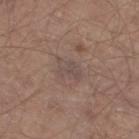* biopsy status — imaged on a skin check; not biopsied
* imaging modality — 15 mm crop, total-body photography
* automated metrics — a lesion color around L≈47 a*≈14 b*≈20 in CIELAB and roughly 5 lightness units darker than nearby skin; a border-irregularity rating of about 4/10, a color-variation rating of about 2.5/10, and peripheral color asymmetry of about 1; a detector confidence of about 95 out of 100 that the crop contains a lesion
* size — ≈3.5 mm
* location — the right thigh
* patient — male, in their mid-60s